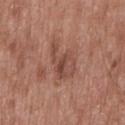Clinical summary:
Measured at roughly 5 mm in maximum diameter. From the mid back. An algorithmic analysis of the crop reported a lesion area of about 9.5 mm² and a symmetry-axis asymmetry near 0.45. The software also gave a border-irregularity rating of about 5.5/10 and internal color variation of about 5.5 on a 0–10 scale. The software also gave an automated nevus-likeness rating near 0 out of 100 and lesion-presence confidence of about 100/100. A roughly 15 mm field-of-view crop from a total-body skin photograph. The patient is a male aged approximately 50.